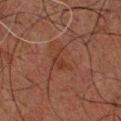Assessment: The lesion was tiled from a total-body skin photograph and was not biopsied. Image and clinical context: The patient is a male aged 58 to 62. The lesion is on the front of the torso. A close-up tile cropped from a whole-body skin photograph, about 15 mm across. The recorded lesion diameter is about 3 mm.